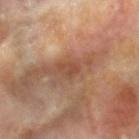Captured during whole-body skin photography for melanoma surveillance; the lesion was not biopsied. This is a cross-polarized tile. The lesion is on the right forearm. A female patient approximately 75 years of age. A region of skin cropped from a whole-body photographic capture, roughly 15 mm wide. The recorded lesion diameter is about 2.5 mm.